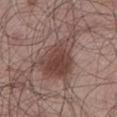| key | value |
|---|---|
| workup | no biopsy performed (imaged during a skin exam) |
| lesion diameter | ≈7.5 mm |
| acquisition | ~15 mm crop, total-body skin-cancer survey |
| location | the left lower leg |
| subject | male, aged 53 to 57 |
| tile lighting | white-light |